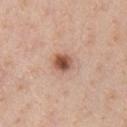Imaged during a routine full-body skin examination; the lesion was not biopsied and no histopathology is available. A roughly 15 mm field-of-view crop from a total-body skin photograph. Captured under white-light illumination. From the chest. The lesion's longest dimension is about 2.5 mm. A male subject, roughly 40 years of age. The lesion-visualizer software estimated an area of roughly 5 mm², an eccentricity of roughly 0.4, and a shape-asymmetry score of about 0.15 (0 = symmetric).